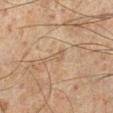notes: imaged on a skin check; not biopsied
illumination: cross-polarized illumination
patient: male, in their mid-40s
image-analysis metrics: a footprint of about 2.5 mm², an outline eccentricity of about 0.95 (0 = round, 1 = elongated), and a shape-asymmetry score of about 0.45 (0 = symmetric); a lesion color around L≈46 a*≈14 b*≈27 in CIELAB and a normalized border contrast of about 4.5; a border-irregularity index near 5/10 and radial color variation of about 0
lesion size: ≈3 mm
location: the left lower leg
imaging modality: total-body-photography crop, ~15 mm field of view